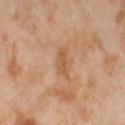Q: What is the lesion's diameter?
A: about 2.5 mm
Q: What kind of image is this?
A: ~15 mm tile from a whole-body skin photo
Q: What lighting was used for the tile?
A: cross-polarized illumination
Q: What is the anatomic site?
A: the leg
Q: What are the patient's age and sex?
A: female, aged approximately 55